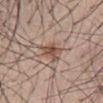The lesion was tiled from a total-body skin photograph and was not biopsied. A 15 mm close-up extracted from a 3D total-body photography capture. The patient is a male in their mid-30s. The lesion is on the abdomen. The recorded lesion diameter is about 4 mm.